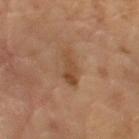Impression: No biopsy was performed on this lesion — it was imaged during a full skin examination and was not determined to be concerning. Clinical summary: Measured at roughly 3.5 mm in maximum diameter. The lesion-visualizer software estimated a mean CIELAB color near L≈45 a*≈19 b*≈32, roughly 8 lightness units darker than nearby skin, and a normalized lesion–skin contrast near 7. And it measured a border-irregularity index near 3.5/10 and a within-lesion color-variation index near 3/10. The software also gave a classifier nevus-likeness of about 20/100. The patient is a male aged 63 to 67. Cropped from a total-body skin-imaging series; the visible field is about 15 mm. Imaged with cross-polarized lighting.